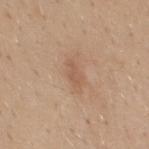Q: What kind of image is this?
A: ~15 mm crop, total-body skin-cancer survey
Q: Patient demographics?
A: male, approximately 40 years of age
Q: Where on the body is the lesion?
A: the mid back